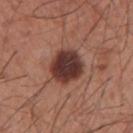Imaged during a routine full-body skin examination; the lesion was not biopsied and no histopathology is available. The recorded lesion diameter is about 4 mm. Imaged with white-light lighting. Automated tile analysis of the lesion measured a lesion area of about 13 mm², an outline eccentricity of about 0.5 (0 = round, 1 = elongated), and two-axis asymmetry of about 0.15. From the right upper arm. A 15 mm close-up extracted from a 3D total-body photography capture. A male subject, approximately 60 years of age.